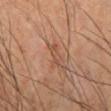Assessment: Captured during whole-body skin photography for melanoma surveillance; the lesion was not biopsied. Context: About 3 mm across. A 15 mm close-up extracted from a 3D total-body photography capture. A male subject approximately 50 years of age. The lesion is located on the right lower leg.